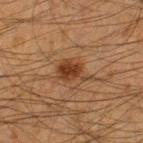{
  "automated_metrics": {
    "color_variation_0_10": 3.5,
    "peripheral_color_asymmetry": 1.5
  },
  "lesion_size": {
    "long_diameter_mm_approx": 4.0
  },
  "site": "left thigh",
  "patient": {
    "sex": "male",
    "age_approx": 35
  },
  "image": {
    "source": "total-body photography crop",
    "field_of_view_mm": 15
  }
}